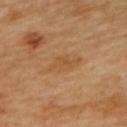The lesion was photographed on a routine skin check and not biopsied; there is no pathology result. A roughly 15 mm field-of-view crop from a total-body skin photograph. Located on the upper back. The patient is a female aged approximately 60.